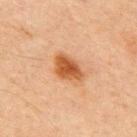Impression: No biopsy was performed on this lesion — it was imaged during a full skin examination and was not determined to be concerning.